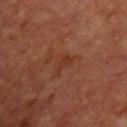This lesion was catalogued during total-body skin photography and was not selected for biopsy. On the upper back. This is a cross-polarized tile. A close-up tile cropped from a whole-body skin photograph, about 15 mm across. A male patient, approximately 65 years of age. An algorithmic analysis of the crop reported a lesion area of about 4 mm², a shape eccentricity near 0.8, and a symmetry-axis asymmetry near 0.5. It also reported a lesion color around L≈32 a*≈22 b*≈28 in CIELAB, roughly 5 lightness units darker than nearby skin, and a lesion-to-skin contrast of about 5 (normalized; higher = more distinct). The software also gave a border-irregularity index near 5/10, a within-lesion color-variation index near 1.5/10, and peripheral color asymmetry of about 0.5. Approximately 3 mm at its widest.